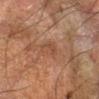Case summary:
• follow-up — total-body-photography surveillance lesion; no biopsy
• patient — male, aged 48 to 52
• size — about 4 mm
• imaging modality — 15 mm crop, total-body photography
• anatomic site — the left forearm
• lighting — cross-polarized
• automated metrics — a lesion area of about 5.5 mm², a shape eccentricity near 0.9, and a shape-asymmetry score of about 0.25 (0 = symmetric); a nevus-likeness score of about 0/100 and lesion-presence confidence of about 85/100